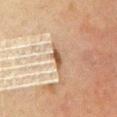Findings:
• diameter · ~3 mm (longest diameter)
• location · the chest
• TBP lesion metrics · a border-irregularity index near 3/10 and internal color variation of about 1.5 on a 0–10 scale; a nevus-likeness score of about 0/100 and lesion-presence confidence of about 90/100
• acquisition · 15 mm crop, total-body photography
• patient · female, aged 58 to 62